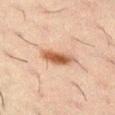| feature | finding |
|---|---|
| follow-up | no biopsy performed (imaged during a skin exam) |
| site | the left thigh |
| image | total-body-photography crop, ~15 mm field of view |
| patient | male, aged around 50 |